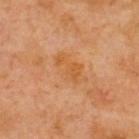notes — no biopsy performed (imaged during a skin exam) | lesion diameter — ≈4.5 mm | acquisition — total-body-photography crop, ~15 mm field of view | subject — male, roughly 70 years of age | anatomic site — the upper back | automated metrics — an area of roughly 8.5 mm², an eccentricity of roughly 0.85, and two-axis asymmetry of about 0.2; a border-irregularity rating of about 2.5/10, a color-variation rating of about 3/10, and radial color variation of about 1; a nevus-likeness score of about 0/100 | tile lighting — cross-polarized.